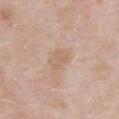<record>
<biopsy_status>not biopsied; imaged during a skin examination</biopsy_status>
<lesion_size>
  <long_diameter_mm_approx>3.5</long_diameter_mm_approx>
</lesion_size>
<patient>
  <sex>male</sex>
  <age_approx>55</age_approx>
</patient>
<image>
  <source>total-body photography crop</source>
  <field_of_view_mm>15</field_of_view_mm>
</image>
<lighting>white-light</lighting>
<site>abdomen</site>
</record>